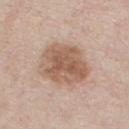The lesion was photographed on a routine skin check and not biopsied; there is no pathology result.